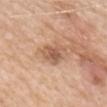Clinical impression: No biopsy was performed on this lesion — it was imaged during a full skin examination and was not determined to be concerning. Acquisition and patient details: Imaged with white-light lighting. The subject is a female approximately 70 years of age. On the head or neck. The lesion-visualizer software estimated a lesion–skin lightness drop of about 11 and a lesion-to-skin contrast of about 7 (normalized; higher = more distinct). It also reported a color-variation rating of about 3.5/10 and peripheral color asymmetry of about 1.5. It also reported a classifier nevus-likeness of about 15/100. The recorded lesion diameter is about 3 mm. A lesion tile, about 15 mm wide, cut from a 3D total-body photograph.